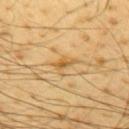| field | value |
|---|---|
| workup | catalogued during a skin exam; not biopsied |
| lighting | cross-polarized |
| patient | male, aged approximately 65 |
| lesion size | about 2.5 mm |
| TBP lesion metrics | an outline eccentricity of about 0.75 (0 = round, 1 = elongated); border irregularity of about 5 on a 0–10 scale and peripheral color asymmetry of about 1; an automated nevus-likeness rating near 0 out of 100 and a detector confidence of about 50 out of 100 that the crop contains a lesion |
| body site | the back |
| imaging modality | total-body-photography crop, ~15 mm field of view |